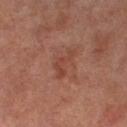This lesion was catalogued during total-body skin photography and was not selected for biopsy. Measured at roughly 4 mm in maximum diameter. A lesion tile, about 15 mm wide, cut from a 3D total-body photograph. The lesion is located on the right thigh. Captured under cross-polarized illumination. A female subject, aged around 60.